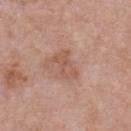The lesion was photographed on a routine skin check and not biopsied; there is no pathology result. The subject is a male about 55 years old. Longest diameter approximately 3.5 mm. Cropped from a total-body skin-imaging series; the visible field is about 15 mm. The lesion is located on the chest. This is a white-light tile.